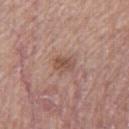Part of a total-body skin-imaging series; this lesion was reviewed on a skin check and was not flagged for biopsy.
Imaged with white-light lighting.
Cropped from a total-body skin-imaging series; the visible field is about 15 mm.
An algorithmic analysis of the crop reported an average lesion color of about L≈52 a*≈20 b*≈26 (CIELAB) and a normalized lesion–skin contrast near 6. And it measured border irregularity of about 2.5 on a 0–10 scale, a within-lesion color-variation index near 4/10, and radial color variation of about 1.
The lesion is located on the right thigh.
About 2.5 mm across.
A male patient approximately 65 years of age.